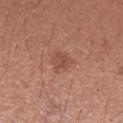The lesion was photographed on a routine skin check and not biopsied; there is no pathology result.
Imaged with white-light lighting.
The lesion is located on the arm.
The lesion's longest dimension is about 2.5 mm.
The subject is a female aged approximately 25.
The lesion-visualizer software estimated an area of roughly 5 mm² and two-axis asymmetry of about 0.25. And it measured a mean CIELAB color near L≈49 a*≈24 b*≈28, a lesion–skin lightness drop of about 7, and a lesion-to-skin contrast of about 5.5 (normalized; higher = more distinct). It also reported a border-irregularity index near 2.5/10 and a peripheral color-asymmetry measure near 1. It also reported an automated nevus-likeness rating near 20 out of 100 and a lesion-detection confidence of about 100/100.
A region of skin cropped from a whole-body photographic capture, roughly 15 mm wide.